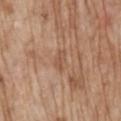Notes:
• biopsy status — catalogued during a skin exam; not biopsied
• image — ~15 mm crop, total-body skin-cancer survey
• patient — male, in their 70s
• anatomic site — the mid back